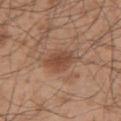Imaged during a routine full-body skin examination; the lesion was not biopsied and no histopathology is available.
Captured under white-light illumination.
About 4.5 mm across.
An algorithmic analysis of the crop reported an outline eccentricity of about 0.85 (0 = round, 1 = elongated). The analysis additionally found a classifier nevus-likeness of about 75/100 and a lesion-detection confidence of about 100/100.
A male patient aged 43 to 47.
A 15 mm close-up tile from a total-body photography series done for melanoma screening.
From the upper back.